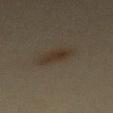Notes:
– follow-up — catalogued during a skin exam; not biopsied
– lesion diameter — ~3.5 mm (longest diameter)
– image — ~15 mm crop, total-body skin-cancer survey
– site — the mid back
– tile lighting — cross-polarized illumination
– subject — female, approximately 45 years of age